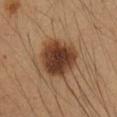{
  "biopsy_status": "not biopsied; imaged during a skin examination",
  "patient": {
    "sex": "female",
    "age_approx": 35
  },
  "site": "right forearm",
  "image": {
    "source": "total-body photography crop",
    "field_of_view_mm": 15
  }
}